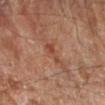Captured during whole-body skin photography for melanoma surveillance; the lesion was not biopsied. A male subject, in their mid- to late 80s or older. The lesion's longest dimension is about 3 mm. From the right forearm. A 15 mm close-up tile from a total-body photography series done for melanoma screening.